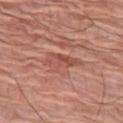Clinical impression: This lesion was catalogued during total-body skin photography and was not selected for biopsy. Context: The subject is a female aged 78–82. A lesion tile, about 15 mm wide, cut from a 3D total-body photograph. The total-body-photography lesion software estimated border irregularity of about 4.5 on a 0–10 scale and radial color variation of about 1. Located on the left thigh. This is a white-light tile.